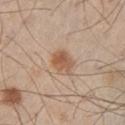Case summary:
– follow-up: imaged on a skin check; not biopsied
– imaging modality: ~15 mm tile from a whole-body skin photo
– size: ≈3.5 mm
– lighting: white-light illumination
– subject: male, approximately 60 years of age
– site: the left thigh